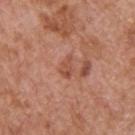The lesion-visualizer software estimated a lesion area of about 3 mm² and a shape-asymmetry score of about 0.35 (0 = symmetric). It also reported a border-irregularity index near 3.5/10, internal color variation of about 2 on a 0–10 scale, and a peripheral color-asymmetry measure near 0.5. The software also gave a classifier nevus-likeness of about 0/100. From the upper back. A male patient, aged 63 to 67. A 15 mm close-up tile from a total-body photography series done for melanoma screening.